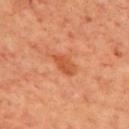Clinical summary:
The subject is a male approximately 70 years of age. The lesion-visualizer software estimated an eccentricity of roughly 0.85 and two-axis asymmetry of about 0.25. The analysis additionally found a mean CIELAB color near L≈52 a*≈30 b*≈41, roughly 9 lightness units darker than nearby skin, and a lesion-to-skin contrast of about 7 (normalized; higher = more distinct). The analysis additionally found border irregularity of about 2.5 on a 0–10 scale, a color-variation rating of about 2/10, and peripheral color asymmetry of about 1. A 15 mm close-up tile from a total-body photography series done for melanoma screening. Located on the back. Approximately 3 mm at its widest.